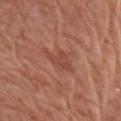The lesion is located on the arm.
A roughly 15 mm field-of-view crop from a total-body skin photograph.
The patient is a female aged approximately 80.
The lesion's longest dimension is about 3.5 mm.